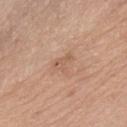Case summary:
– automated metrics — an average lesion color of about L≈59 a*≈19 b*≈31 (CIELAB) and about 7 CIELAB-L* units darker than the surrounding skin; border irregularity of about 5.5 on a 0–10 scale and a within-lesion color-variation index near 2.5/10; a nevus-likeness score of about 0/100 and a detector confidence of about 100 out of 100 that the crop contains a lesion
– anatomic site — the abdomen
– illumination — white-light illumination
– patient — female, approximately 60 years of age
– lesion diameter — ≈3 mm
– acquisition — ~15 mm tile from a whole-body skin photo